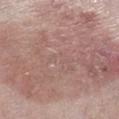notes=no biopsy performed (imaged during a skin exam).